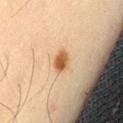The lesion was tiled from a total-body skin photograph and was not biopsied.
A male patient approximately 65 years of age.
About 2.5 mm across.
The tile uses cross-polarized illumination.
A 15 mm close-up tile from a total-body photography series done for melanoma screening.
On the lower back.